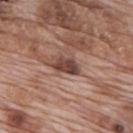Part of a total-body skin-imaging series; this lesion was reviewed on a skin check and was not flagged for biopsy. A male subject, roughly 70 years of age. Approximately 3.5 mm at its widest. A 15 mm close-up extracted from a 3D total-body photography capture. The total-body-photography lesion software estimated about 15 CIELAB-L* units darker than the surrounding skin and a normalized lesion–skin contrast near 11. And it measured a border-irregularity index near 3/10 and internal color variation of about 4.5 on a 0–10 scale. It also reported a classifier nevus-likeness of about 40/100 and lesion-presence confidence of about 95/100. The lesion is on the upper back. The tile uses white-light illumination.